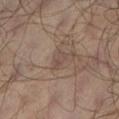Assessment:
This lesion was catalogued during total-body skin photography and was not selected for biopsy.
Context:
This image is a 15 mm lesion crop taken from a total-body photograph. Imaged with cross-polarized lighting. From the left lower leg. The recorded lesion diameter is about 2.5 mm. A male patient, in their mid-60s.